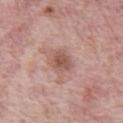The lesion was tiled from a total-body skin photograph and was not biopsied. On the abdomen. The patient is a male aged 68–72. A 15 mm close-up extracted from a 3D total-body photography capture. The recorded lesion diameter is about 3 mm.